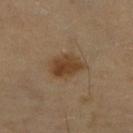<record>
<biopsy_status>not biopsied; imaged during a skin examination</biopsy_status>
<automated_metrics>
  <area_mm2_approx>9.0</area_mm2_approx>
  <eccentricity>0.8</eccentricity>
  <shape_asymmetry>0.2</shape_asymmetry>
  <cielab_L>42</cielab_L>
  <cielab_a>16</cielab_a>
  <cielab_b>32</cielab_b>
  <vs_skin_darker_L>10.0</vs_skin_darker_L>
  <vs_skin_contrast_norm>9.0</vs_skin_contrast_norm>
</automated_metrics>
<lighting>cross-polarized</lighting>
<image>
  <source>total-body photography crop</source>
  <field_of_view_mm>15</field_of_view_mm>
</image>
<site>right lower leg</site>
<patient>
  <sex>female</sex>
  <age_approx>70</age_approx>
</patient>
</record>